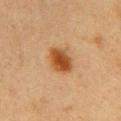workup=imaged on a skin check; not biopsied | subject=female, aged approximately 50 | size=~3.5 mm (longest diameter) | TBP lesion metrics=an average lesion color of about L≈42 a*≈22 b*≈36 (CIELAB), about 12 CIELAB-L* units darker than the surrounding skin, and a normalized border contrast of about 10.5; a border-irregularity rating of about 1.5/10 and radial color variation of about 1.5; lesion-presence confidence of about 100/100 | location=the chest | image source=total-body-photography crop, ~15 mm field of view.